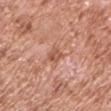Recorded during total-body skin imaging; not selected for excision or biopsy. Automated image analysis of the tile measured a border-irregularity index near 3/10 and peripheral color asymmetry of about 1. The software also gave an automated nevus-likeness rating near 0 out of 100 and a detector confidence of about 100 out of 100 that the crop contains a lesion. The subject is a male in their mid- to late 60s. A close-up tile cropped from a whole-body skin photograph, about 15 mm across. On the left upper arm. Imaged with white-light lighting. Measured at roughly 3 mm in maximum diameter.